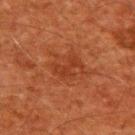Assessment: This lesion was catalogued during total-body skin photography and was not selected for biopsy. Context: The total-body-photography lesion software estimated a lesion color around L≈30 a*≈25 b*≈30 in CIELAB, about 5 CIELAB-L* units darker than the surrounding skin, and a lesion-to-skin contrast of about 5.5 (normalized; higher = more distinct). The software also gave a border-irregularity rating of about 7/10, a color-variation rating of about 1/10, and peripheral color asymmetry of about 0. Located on the upper back. A roughly 15 mm field-of-view crop from a total-body skin photograph. Captured under cross-polarized illumination. A male subject aged 58 to 62.